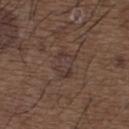| field | value |
|---|---|
| notes | total-body-photography surveillance lesion; no biopsy |
| location | the upper back |
| lesion size | about 3 mm |
| acquisition | 15 mm crop, total-body photography |
| subject | male, aged approximately 50 |
| automated metrics | a lesion area of about 6.5 mm² and a symmetry-axis asymmetry near 0.25; a lesion color around L≈35 a*≈15 b*≈20 in CIELAB and a normalized lesion–skin contrast near 5; a within-lesion color-variation index near 3/10 |
| illumination | white-light illumination |